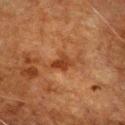Q: Was a biopsy performed?
A: total-body-photography surveillance lesion; no biopsy
Q: Automated lesion metrics?
A: an area of roughly 4 mm², an outline eccentricity of about 0.75 (0 = round, 1 = elongated), and a symmetry-axis asymmetry near 0.45; a lesion color around L≈32 a*≈23 b*≈31 in CIELAB, roughly 8 lightness units darker than nearby skin, and a normalized lesion–skin contrast near 8; a border-irregularity index near 4.5/10, internal color variation of about 2.5 on a 0–10 scale, and peripheral color asymmetry of about 1
Q: How was this image acquired?
A: 15 mm crop, total-body photography
Q: Who is the patient?
A: male, aged approximately 80
Q: What is the lesion's diameter?
A: ~3 mm (longest diameter)
Q: What is the anatomic site?
A: the chest
Q: Illumination type?
A: cross-polarized